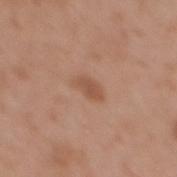Q: Is there a histopathology result?
A: total-body-photography surveillance lesion; no biopsy
Q: What are the patient's age and sex?
A: female, approximately 35 years of age
Q: What is the anatomic site?
A: the back
Q: How was this image acquired?
A: ~15 mm tile from a whole-body skin photo
Q: How was the tile lit?
A: white-light
Q: What did automated image analysis measure?
A: an area of roughly 4.5 mm², a shape eccentricity near 0.85, and a symmetry-axis asymmetry near 0.25; a lesion color around L≈53 a*≈21 b*≈31 in CIELAB, about 8 CIELAB-L* units darker than the surrounding skin, and a normalized lesion–skin contrast near 6; a border-irregularity rating of about 2.5/10, a within-lesion color-variation index near 1.5/10, and peripheral color asymmetry of about 0.5; an automated nevus-likeness rating near 15 out of 100
Q: Lesion size?
A: ~3 mm (longest diameter)